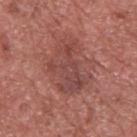Clinical impression:
The lesion was tiled from a total-body skin photograph and was not biopsied.
Clinical summary:
The lesion is located on the upper back. Captured under white-light illumination. An algorithmic analysis of the crop reported a lesion area of about 17 mm², an outline eccentricity of about 0.75 (0 = round, 1 = elongated), and a symmetry-axis asymmetry near 0.45. And it measured an average lesion color of about L≈45 a*≈25 b*≈24 (CIELAB), roughly 7 lightness units darker than nearby skin, and a normalized border contrast of about 6. And it measured a border-irregularity rating of about 7.5/10, a color-variation rating of about 4/10, and peripheral color asymmetry of about 1.5. And it measured a classifier nevus-likeness of about 0/100 and a lesion-detection confidence of about 100/100. A roughly 15 mm field-of-view crop from a total-body skin photograph. The lesion's longest dimension is about 6 mm. A male subject about 75 years old.